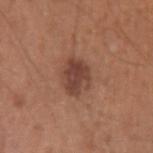Imaged during a routine full-body skin examination; the lesion was not biopsied and no histopathology is available. Measured at roughly 3.5 mm in maximum diameter. On the left upper arm. Cropped from a total-body skin-imaging series; the visible field is about 15 mm. Captured under white-light illumination. A male patient in their mid- to late 30s.